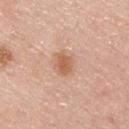Longest diameter approximately 3 mm.
A 15 mm close-up tile from a total-body photography series done for melanoma screening.
Captured under white-light illumination.
A male subject, roughly 45 years of age.
The lesion is located on the chest.